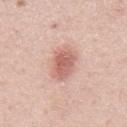Q: Was this lesion biopsied?
A: total-body-photography surveillance lesion; no biopsy
Q: Who is the patient?
A: male, in their mid- to late 50s
Q: What is the lesion's diameter?
A: ≈4.5 mm
Q: How was the tile lit?
A: white-light
Q: How was this image acquired?
A: total-body-photography crop, ~15 mm field of view
Q: What is the anatomic site?
A: the mid back